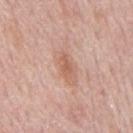Impression:
Imaged during a routine full-body skin examination; the lesion was not biopsied and no histopathology is available.
Image and clinical context:
A male patient, in their mid-70s. The lesion is located on the mid back. A region of skin cropped from a whole-body photographic capture, roughly 15 mm wide.